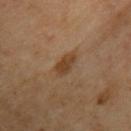follow-up = total-body-photography surveillance lesion; no biopsy | patient = male, aged around 70 | location = the left upper arm | acquisition = ~15 mm crop, total-body skin-cancer survey | tile lighting = cross-polarized illumination.